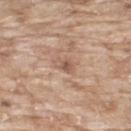This is a white-light tile.
The recorded lesion diameter is about 2.5 mm.
Located on the upper back.
A 15 mm crop from a total-body photograph taken for skin-cancer surveillance.
A male patient roughly 65 years of age.